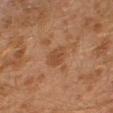Recorded during total-body skin imaging; not selected for excision or biopsy. This image is a 15 mm lesion crop taken from a total-body photograph. The lesion-visualizer software estimated a footprint of about 4 mm², an eccentricity of roughly 0.6, and a shape-asymmetry score of about 0.25 (0 = symmetric). The software also gave a mean CIELAB color near L≈46 a*≈22 b*≈34, roughly 7 lightness units darker than nearby skin, and a normalized border contrast of about 6. Measured at roughly 2.5 mm in maximum diameter. On the left leg. A male subject, in their 30s.